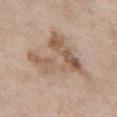Notes:
– notes — no biopsy performed (imaged during a skin exam)
– diameter — ~8 mm (longest diameter)
– image — ~15 mm tile from a whole-body skin photo
– lighting — white-light illumination
– automated lesion analysis — an area of roughly 23 mm², a shape eccentricity near 0.75, and two-axis asymmetry of about 0.65; an average lesion color of about L≈58 a*≈16 b*≈28 (CIELAB) and about 10 CIELAB-L* units darker than the surrounding skin; border irregularity of about 8.5 on a 0–10 scale and a color-variation rating of about 7/10; a classifier nevus-likeness of about 15/100 and a detector confidence of about 85 out of 100 that the crop contains a lesion
– patient — female, aged around 75
– site — the chest